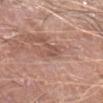The lesion was tiled from a total-body skin photograph and was not biopsied.
Approximately 3 mm at its widest.
The lesion is on the left forearm.
A region of skin cropped from a whole-body photographic capture, roughly 15 mm wide.
This is a white-light tile.
The lesion-visualizer software estimated a footprint of about 4.5 mm² and a shape-asymmetry score of about 0.35 (0 = symmetric). The analysis additionally found a border-irregularity rating of about 4/10, a within-lesion color-variation index near 4/10, and radial color variation of about 1.5. And it measured a nevus-likeness score of about 0/100.
A male subject, aged 63 to 67.